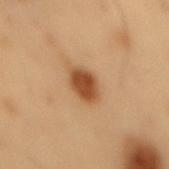Q: How was this image acquired?
A: total-body-photography crop, ~15 mm field of view
Q: What are the patient's age and sex?
A: male, in their mid- to late 50s
Q: Lesion location?
A: the mid back